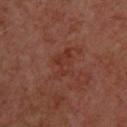notes: no biopsy performed (imaged during a skin exam)
tile lighting: cross-polarized
patient: male, aged approximately 50
diameter: ~3.5 mm (longest diameter)
image: ~15 mm tile from a whole-body skin photo
body site: the upper back